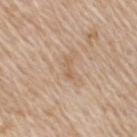{
  "biopsy_status": "not biopsied; imaged during a skin examination",
  "site": "upper back",
  "patient": {
    "sex": "male",
    "age_approx": 60
  },
  "image": {
    "source": "total-body photography crop",
    "field_of_view_mm": 15
  },
  "lighting": "white-light",
  "lesion_size": {
    "long_diameter_mm_approx": 2.5
  }
}